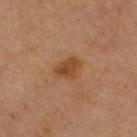Q: Is there a histopathology result?
A: imaged on a skin check; not biopsied
Q: What is the imaging modality?
A: total-body-photography crop, ~15 mm field of view
Q: Lesion location?
A: the arm